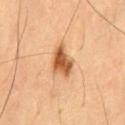diameter: ~4 mm (longest diameter) | subject: male, about 60 years old | acquisition: 15 mm crop, total-body photography | site: the lower back | automated metrics: a lesion area of about 8 mm² and a shape-asymmetry score of about 0.3 (0 = symmetric); a mean CIELAB color near L≈58 a*≈24 b*≈41, about 16 CIELAB-L* units darker than the surrounding skin, and a normalized border contrast of about 10; a border-irregularity index near 3/10 | lighting: cross-polarized illumination.